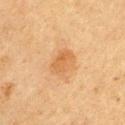Clinical impression: This lesion was catalogued during total-body skin photography and was not selected for biopsy.